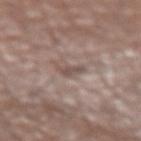Notes:
– biopsy status — imaged on a skin check; not biopsied
– lighting — white-light
– acquisition — ~15 mm tile from a whole-body skin photo
– diameter — ~2.5 mm (longest diameter)
– subject — male, aged 73–77
– anatomic site — the arm